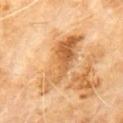Clinical impression: This lesion was catalogued during total-body skin photography and was not selected for biopsy. Acquisition and patient details: The subject is a male aged 58 to 62. Cropped from a whole-body photographic skin survey; the tile spans about 15 mm. On the chest.